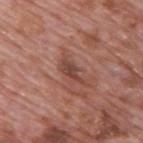{
  "biopsy_status": "not biopsied; imaged during a skin examination",
  "site": "upper back",
  "image": {
    "source": "total-body photography crop",
    "field_of_view_mm": 15
  },
  "lesion_size": {
    "long_diameter_mm_approx": 4.0
  },
  "patient": {
    "sex": "male",
    "age_approx": 70
  }
}